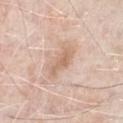This lesion was catalogued during total-body skin photography and was not selected for biopsy.
This image is a 15 mm lesion crop taken from a total-body photograph.
The lesion is on the chest.
The subject is a male aged 73–77.